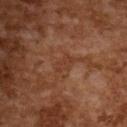The lesion was photographed on a routine skin check and not biopsied; there is no pathology result.